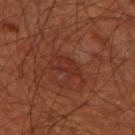Part of a total-body skin-imaging series; this lesion was reviewed on a skin check and was not flagged for biopsy. The lesion is on the left thigh. A male patient, roughly 70 years of age. A 15 mm close-up tile from a total-body photography series done for melanoma screening.